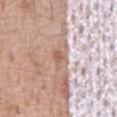| field | value |
|---|---|
| size | about 3 mm |
| subject | male, aged 58 to 62 |
| tile lighting | white-light illumination |
| image source | ~15 mm crop, total-body skin-cancer survey |
| site | the abdomen |
| image-analysis metrics | a shape eccentricity near 0.8; a nevus-likeness score of about 0/100 |